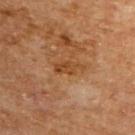This lesion was catalogued during total-body skin photography and was not selected for biopsy.
About 3.5 mm across.
A lesion tile, about 15 mm wide, cut from a 3D total-body photograph.
A male subject, in their mid- to late 60s.
Captured under cross-polarized illumination.
Located on the upper back.